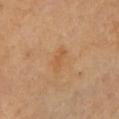This lesion was catalogued during total-body skin photography and was not selected for biopsy. This image is a 15 mm lesion crop taken from a total-body photograph. Located on the front of the torso. An algorithmic analysis of the crop reported a lesion area of about 4 mm² and two-axis asymmetry of about 0.35. The analysis additionally found an average lesion color of about L≈57 a*≈21 b*≈39 (CIELAB), a lesion–skin lightness drop of about 6, and a normalized lesion–skin contrast near 5. And it measured an automated nevus-likeness rating near 0 out of 100. A female subject, about 70 years old.